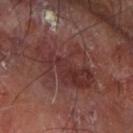notes=no biopsy performed (imaged during a skin exam)
illumination=cross-polarized illumination
subject=male, in their mid-60s
lesion size=~7 mm (longest diameter)
image source=~15 mm tile from a whole-body skin photo
location=the left forearm